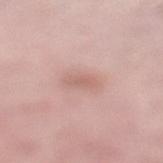follow-up = no biopsy performed (imaged during a skin exam).